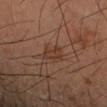The subject is a male aged approximately 65.
Approximately 3.5 mm at its widest.
A lesion tile, about 15 mm wide, cut from a 3D total-body photograph.
The total-body-photography lesion software estimated border irregularity of about 4.5 on a 0–10 scale, a color-variation rating of about 2/10, and radial color variation of about 0.5. It also reported an automated nevus-likeness rating near 40 out of 100 and lesion-presence confidence of about 100/100.
The lesion is on the left forearm.